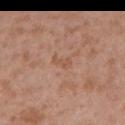Assessment: Captured during whole-body skin photography for melanoma surveillance; the lesion was not biopsied. Clinical summary: Imaged with white-light lighting. A region of skin cropped from a whole-body photographic capture, roughly 15 mm wide. The lesion-visualizer software estimated a color-variation rating of about 0/10 and peripheral color asymmetry of about 0. And it measured an automated nevus-likeness rating near 0 out of 100 and a detector confidence of about 100 out of 100 that the crop contains a lesion. On the left upper arm. The patient is a male aged 38 to 42.